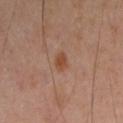This lesion was catalogued during total-body skin photography and was not selected for biopsy.
From the right upper arm.
A region of skin cropped from a whole-body photographic capture, roughly 15 mm wide.
Automated image analysis of the tile measured a nevus-likeness score of about 85/100.
The patient is a male in their mid- to late 40s.
Imaged with cross-polarized lighting.
Measured at roughly 2.5 mm in maximum diameter.